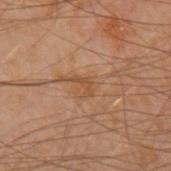Impression:
Captured during whole-body skin photography for melanoma surveillance; the lesion was not biopsied.
Acquisition and patient details:
Imaged with cross-polarized lighting. Located on the left forearm. A 15 mm close-up extracted from a 3D total-body photography capture. An algorithmic analysis of the crop reported an area of roughly 3 mm² and a shape eccentricity near 0.8. The software also gave an automated nevus-likeness rating near 0 out of 100 and lesion-presence confidence of about 100/100. A male subject, aged 48–52. Approximately 2.5 mm at its widest.